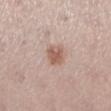| key | value |
|---|---|
| image source | ~15 mm crop, total-body skin-cancer survey |
| site | the left lower leg |
| lesion size | ~2.5 mm (longest diameter) |
| patient | female, approximately 40 years of age |
| lighting | white-light |